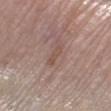notes = catalogued during a skin exam; not biopsied
image-analysis metrics = a footprint of about 3 mm² and an eccentricity of roughly 0.95; an average lesion color of about L≈51 a*≈18 b*≈24 (CIELAB), about 7 CIELAB-L* units darker than the surrounding skin, and a normalized border contrast of about 5.5; a nevus-likeness score of about 0/100
location = the right lower leg
tile lighting = white-light
lesion diameter = ≈3 mm
subject = female, about 65 years old
acquisition = ~15 mm tile from a whole-body skin photo